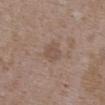Clinical impression: Recorded during total-body skin imaging; not selected for excision or biopsy. Context: Located on the upper back. The subject is a female in their mid-30s. A 15 mm close-up tile from a total-body photography series done for melanoma screening.